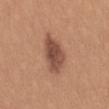Q: Was this lesion biopsied?
A: imaged on a skin check; not biopsied
Q: What is the imaging modality?
A: ~15 mm crop, total-body skin-cancer survey
Q: Automated lesion metrics?
A: an outline eccentricity of about 0.85 (0 = round, 1 = elongated) and a shape-asymmetry score of about 0.25 (0 = symmetric); an average lesion color of about L≈49 a*≈22 b*≈28 (CIELAB) and a normalized lesion–skin contrast near 9; an automated nevus-likeness rating near 80 out of 100 and a lesion-detection confidence of about 100/100
Q: How was the tile lit?
A: white-light
Q: Where on the body is the lesion?
A: the mid back
Q: Lesion size?
A: ~5.5 mm (longest diameter)
Q: Who is the patient?
A: female, aged 38 to 42